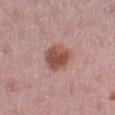Q: Was a biopsy performed?
A: catalogued during a skin exam; not biopsied
Q: What did automated image analysis measure?
A: a lesion area of about 9.5 mm², an outline eccentricity of about 0.6 (0 = round, 1 = elongated), and two-axis asymmetry of about 0.2; a mean CIELAB color near L≈51 a*≈23 b*≈26; an automated nevus-likeness rating near 95 out of 100 and lesion-presence confidence of about 100/100
Q: Lesion size?
A: about 4 mm
Q: What kind of image is this?
A: total-body-photography crop, ~15 mm field of view
Q: Where on the body is the lesion?
A: the left lower leg
Q: Who is the patient?
A: female, aged 43–47
Q: Illumination type?
A: white-light